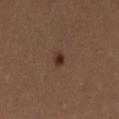| feature | finding |
|---|---|
| follow-up | catalogued during a skin exam; not biopsied |
| anatomic site | the leg |
| size | ~2.5 mm (longest diameter) |
| image-analysis metrics | a lesion area of about 2.5 mm², an eccentricity of roughly 0.8, and a symmetry-axis asymmetry near 0.35; a lesion color around L≈29 a*≈18 b*≈24 in CIELAB and a normalized lesion–skin contrast near 9.5; border irregularity of about 3 on a 0–10 scale, internal color variation of about 1.5 on a 0–10 scale, and peripheral color asymmetry of about 0.5 |
| illumination | cross-polarized illumination |
| imaging modality | total-body-photography crop, ~15 mm field of view |
| patient | female, aged 63 to 67 |